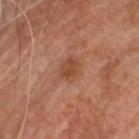- biopsy status · imaged on a skin check; not biopsied
- site · the head or neck
- lesion diameter · ≈3 mm
- image · ~15 mm tile from a whole-body skin photo
- tile lighting · cross-polarized illumination
- image-analysis metrics · an area of roughly 5 mm² and two-axis asymmetry of about 0.2; a lesion color around L≈45 a*≈23 b*≈31 in CIELAB, roughly 8 lightness units darker than nearby skin, and a normalized border contrast of about 6.5; a border-irregularity rating of about 2/10, internal color variation of about 3 on a 0–10 scale, and peripheral color asymmetry of about 1; a classifier nevus-likeness of about 20/100 and lesion-presence confidence of about 100/100
- patient · male, roughly 70 years of age